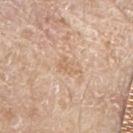Clinical impression: No biopsy was performed on this lesion — it was imaged during a full skin examination and was not determined to be concerning. Clinical summary: Approximately 2.5 mm at its widest. On the right upper arm. Imaged with white-light lighting. This image is a 15 mm lesion crop taken from a total-body photograph. A male patient aged 78–82.